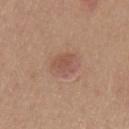This lesion was catalogued during total-body skin photography and was not selected for biopsy. A 15 mm crop from a total-body photograph taken for skin-cancer surveillance. From the mid back. This is a white-light tile. A female patient, aged 33–37.